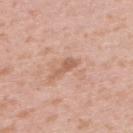<tbp_lesion>
  <biopsy_status>not biopsied; imaged during a skin examination</biopsy_status>
  <lighting>white-light</lighting>
  <site>upper back</site>
  <patient>
    <sex>female</sex>
    <age_approx>40</age_approx>
  </patient>
  <lesion_size>
    <long_diameter_mm_approx>3.0</long_diameter_mm_approx>
  </lesion_size>
  <automated_metrics>
    <border_irregularity_0_10>5.0</border_irregularity_0_10>
    <peripheral_color_asymmetry>0.0</peripheral_color_asymmetry>
  </automated_metrics>
  <image>
    <source>total-body photography crop</source>
    <field_of_view_mm>15</field_of_view_mm>
  </image>
</tbp_lesion>